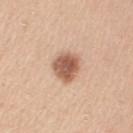biopsy status: no biopsy performed (imaged during a skin exam) | acquisition: total-body-photography crop, ~15 mm field of view | patient: female, aged approximately 45 | location: the left upper arm | automated metrics: roughly 16 lightness units darker than nearby skin and a lesion-to-skin contrast of about 10 (normalized; higher = more distinct); a classifier nevus-likeness of about 95/100.